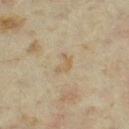Clinical impression: Captured during whole-body skin photography for melanoma surveillance; the lesion was not biopsied. Background: Cropped from a whole-body photographic skin survey; the tile spans about 15 mm. The lesion-visualizer software estimated a border-irregularity rating of about 5/10 and a peripheral color-asymmetry measure near 0.5. The analysis additionally found a nevus-likeness score of about 0/100 and a lesion-detection confidence of about 100/100. A female subject, approximately 35 years of age. From the left thigh. Captured under cross-polarized illumination.